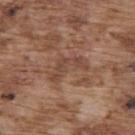Notes:
* workup · catalogued during a skin exam; not biopsied
* acquisition · ~15 mm crop, total-body skin-cancer survey
* body site · the back
* subject · male, aged 73 to 77
* automated metrics · a mean CIELAB color near L≈45 a*≈20 b*≈27; a classifier nevus-likeness of about 0/100 and lesion-presence confidence of about 70/100
* diameter · ≈5 mm
* lighting · white-light illumination